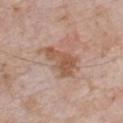Notes:
- follow-up · imaged on a skin check; not biopsied